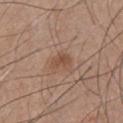{"image": {"source": "total-body photography crop", "field_of_view_mm": 15}, "lighting": "white-light", "site": "chest", "patient": {"sex": "male", "age_approx": 55}, "lesion_size": {"long_diameter_mm_approx": 2.5}, "automated_metrics": {"cielab_L": 49, "cielab_a": 19, "cielab_b": 30, "vs_skin_darker_L": 8.0, "vs_skin_contrast_norm": 6.5}}